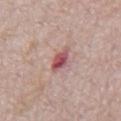Notes:
- workup: total-body-photography surveillance lesion; no biopsy
- image-analysis metrics: a lesion color around L≈52 a*≈28 b*≈19 in CIELAB and a lesion–skin lightness drop of about 14; a border-irregularity index near 2.5/10, a within-lesion color-variation index near 6.5/10, and a peripheral color-asymmetry measure near 2
- location: the abdomen
- lighting: white-light
- lesion diameter: ~3 mm (longest diameter)
- image: total-body-photography crop, ~15 mm field of view
- patient: male, about 70 years old